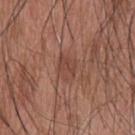This lesion was catalogued during total-body skin photography and was not selected for biopsy. About 3 mm across. A region of skin cropped from a whole-body photographic capture, roughly 15 mm wide. From the upper back. An algorithmic analysis of the crop reported an average lesion color of about L≈44 a*≈22 b*≈26 (CIELAB). Captured under white-light illumination. A male subject, in their mid- to late 40s.